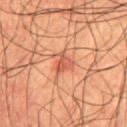The lesion was tiled from a total-body skin photograph and was not biopsied.
Longest diameter approximately 2.5 mm.
A 15 mm close-up extracted from a 3D total-body photography capture.
On the chest.
Imaged with cross-polarized lighting.
A male patient approximately 55 years of age.
Automated tile analysis of the lesion measured roughly 9 lightness units darker than nearby skin and a normalized lesion–skin contrast near 6.5. The software also gave peripheral color asymmetry of about 1. The analysis additionally found a nevus-likeness score of about 25/100 and lesion-presence confidence of about 100/100.